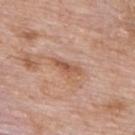Clinical impression:
The lesion was photographed on a routine skin check and not biopsied; there is no pathology result.